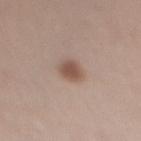{
  "biopsy_status": "not biopsied; imaged during a skin examination",
  "image": {
    "source": "total-body photography crop",
    "field_of_view_mm": 15
  },
  "site": "right thigh",
  "lesion_size": {
    "long_diameter_mm_approx": 3.0
  },
  "patient": {
    "sex": "female",
    "age_approx": 30
  }
}